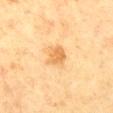Notes:
- notes · no biopsy performed (imaged during a skin exam)
- site · the back
- automated lesion analysis · an eccentricity of roughly 0.65 and two-axis asymmetry of about 0.25; a border-irregularity rating of about 2.5/10 and a within-lesion color-variation index near 2/10; an automated nevus-likeness rating near 55 out of 100 and a detector confidence of about 100 out of 100 that the crop contains a lesion
- patient · female, approximately 50 years of age
- imaging modality · ~15 mm tile from a whole-body skin photo
- lighting · cross-polarized
- diameter · ≈2.5 mm